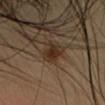Findings:
– follow-up · no biopsy performed (imaged during a skin exam)
– patient · female, aged 33–37
– illumination · cross-polarized
– image · ~15 mm tile from a whole-body skin photo
– location · the head or neck
– lesion diameter · ~3 mm (longest diameter)